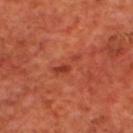This lesion was catalogued during total-body skin photography and was not selected for biopsy. The lesion is on the upper back. A male patient, approximately 70 years of age. The recorded lesion diameter is about 4 mm. Cropped from a total-body skin-imaging series; the visible field is about 15 mm.